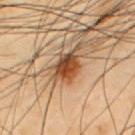Assessment:
Imaged during a routine full-body skin examination; the lesion was not biopsied and no histopathology is available.
Acquisition and patient details:
Cropped from a whole-body photographic skin survey; the tile spans about 15 mm. A male subject about 55 years old. Approximately 3.5 mm at its widest. Located on the chest. Imaged with cross-polarized lighting.